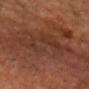workup: total-body-photography surveillance lesion; no biopsy
imaging modality: total-body-photography crop, ~15 mm field of view
lighting: cross-polarized
image-analysis metrics: an area of roughly 18 mm², an outline eccentricity of about 0.85 (0 = round, 1 = elongated), and a symmetry-axis asymmetry near 0.45; a mean CIELAB color near L≈34 a*≈22 b*≈28 and roughly 5 lightness units darker than nearby skin; a border-irregularity rating of about 8/10, internal color variation of about 4 on a 0–10 scale, and radial color variation of about 1
subject: male, aged 68 to 72
anatomic site: the chest
lesion diameter: about 7.5 mm